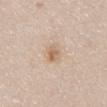Q: Was a biopsy performed?
A: imaged on a skin check; not biopsied
Q: What is the anatomic site?
A: the abdomen
Q: Automated lesion metrics?
A: an eccentricity of roughly 0.75 and a symmetry-axis asymmetry near 0.15; a border-irregularity rating of about 2/10, a within-lesion color-variation index near 3/10, and peripheral color asymmetry of about 1
Q: How was this image acquired?
A: ~15 mm crop, total-body skin-cancer survey
Q: Lesion size?
A: about 2.5 mm
Q: What lighting was used for the tile?
A: white-light
Q: Who is the patient?
A: male, roughly 80 years of age